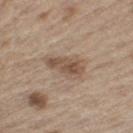Impression:
Recorded during total-body skin imaging; not selected for excision or biopsy.
Image and clinical context:
A male subject aged approximately 70. The lesion is on the left thigh. Automated tile analysis of the lesion measured an area of roughly 7 mm², a shape eccentricity near 0.9, and a shape-asymmetry score of about 0.3 (0 = symmetric). The analysis additionally found a nevus-likeness score of about 5/100 and lesion-presence confidence of about 100/100. This is a white-light tile. A roughly 15 mm field-of-view crop from a total-body skin photograph. About 4 mm across.